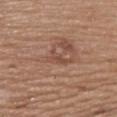Q: Is there a histopathology result?
A: no biopsy performed (imaged during a skin exam)
Q: What are the patient's age and sex?
A: female, aged 63–67
Q: Lesion size?
A: about 3 mm
Q: What did automated image analysis measure?
A: a lesion color around L≈48 a*≈22 b*≈28 in CIELAB and a normalized border contrast of about 5.5; a classifier nevus-likeness of about 0/100 and lesion-presence confidence of about 100/100
Q: What lighting was used for the tile?
A: white-light
Q: Where on the body is the lesion?
A: the upper back
Q: What is the imaging modality?
A: 15 mm crop, total-body photography